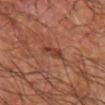Impression: This lesion was catalogued during total-body skin photography and was not selected for biopsy. Context: Located on the left upper arm. A male subject aged around 65. About 3 mm across. A 15 mm close-up tile from a total-body photography series done for melanoma screening. The tile uses cross-polarized illumination.